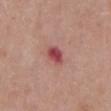Q: Is there a histopathology result?
A: no biopsy performed (imaged during a skin exam)
Q: What lighting was used for the tile?
A: white-light illumination
Q: What is the imaging modality?
A: ~15 mm tile from a whole-body skin photo
Q: Automated lesion metrics?
A: a lesion color around L≈48 a*≈32 b*≈21 in CIELAB and a lesion-to-skin contrast of about 9.5 (normalized; higher = more distinct); a border-irregularity index near 2/10 and internal color variation of about 4 on a 0–10 scale; lesion-presence confidence of about 100/100
Q: Lesion location?
A: the chest
Q: How large is the lesion?
A: ~3 mm (longest diameter)
Q: Who is the patient?
A: female, aged around 65